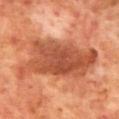Clinical impression: The lesion was tiled from a total-body skin photograph and was not biopsied. Context: The total-body-photography lesion software estimated a mean CIELAB color near L≈50 a*≈29 b*≈36, about 13 CIELAB-L* units darker than the surrounding skin, and a normalized border contrast of about 8.5. The software also gave a border-irregularity index near 5.5/10 and a peripheral color-asymmetry measure near 1.5. The software also gave a classifier nevus-likeness of about 0/100 and lesion-presence confidence of about 100/100. A male subject, about 70 years old. A 15 mm close-up tile from a total-body photography series done for melanoma screening. The lesion is on the back. Imaged with cross-polarized lighting. About 11.5 mm across.